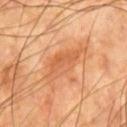{
  "biopsy_status": "not biopsied; imaged during a skin examination",
  "lesion_size": {
    "long_diameter_mm_approx": 6.0
  },
  "site": "chest",
  "image": {
    "source": "total-body photography crop",
    "field_of_view_mm": 15
  },
  "lighting": "cross-polarized",
  "automated_metrics": {
    "cielab_L": 58,
    "cielab_a": 26,
    "cielab_b": 40,
    "vs_skin_darker_L": 8.0,
    "vs_skin_contrast_norm": 5.5,
    "border_irregularity_0_10": 4.0,
    "color_variation_0_10": 4.0,
    "peripheral_color_asymmetry": 1.5
  },
  "patient": {
    "sex": "male",
    "age_approx": 65
  }
}